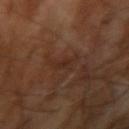Recorded during total-body skin imaging; not selected for excision or biopsy. The tile uses cross-polarized illumination. About 3 mm across. A male patient, in their 70s. The lesion is located on the right upper arm. A close-up tile cropped from a whole-body skin photograph, about 15 mm across.